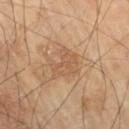Q: Was a biopsy performed?
A: imaged on a skin check; not biopsied
Q: Where on the body is the lesion?
A: the right thigh
Q: Lesion size?
A: ≈3.5 mm
Q: What did automated image analysis measure?
A: an area of roughly 9.5 mm², an eccentricity of roughly 0.4, and a symmetry-axis asymmetry near 0.45; a lesion color around L≈55 a*≈18 b*≈33 in CIELAB, roughly 7 lightness units darker than nearby skin, and a normalized lesion–skin contrast near 4.5; a border-irregularity rating of about 5.5/10, internal color variation of about 2 on a 0–10 scale, and radial color variation of about 1
Q: What kind of image is this?
A: ~15 mm crop, total-body skin-cancer survey
Q: What lighting was used for the tile?
A: cross-polarized illumination
Q: Patient demographics?
A: male, approximately 70 years of age